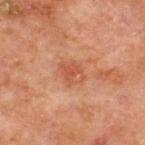Measured at roughly 3 mm in maximum diameter.
The subject is a male roughly 65 years of age.
This image is a 15 mm lesion crop taken from a total-body photograph.
This is a cross-polarized tile.
On the chest.